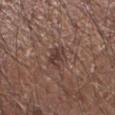This lesion was catalogued during total-body skin photography and was not selected for biopsy.